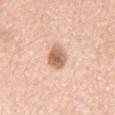follow-up = catalogued during a skin exam; not biopsied | body site = the chest | image source = 15 mm crop, total-body photography | patient = male, roughly 75 years of age | tile lighting = white-light.